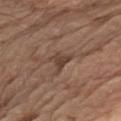Case summary:
• size: ≈3.5 mm
• tile lighting: white-light illumination
• automated lesion analysis: a lesion area of about 5 mm², an outline eccentricity of about 0.55 (0 = round, 1 = elongated), and a shape-asymmetry score of about 0.5 (0 = symmetric); roughly 8 lightness units darker than nearby skin and a normalized border contrast of about 7
• site: the left upper arm
• subject: male, about 70 years old
• image: total-body-photography crop, ~15 mm field of view